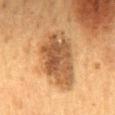workup: no biopsy performed (imaged during a skin exam)
automated metrics: an area of roughly 26 mm², an outline eccentricity of about 0.8 (0 = round, 1 = elongated), and a symmetry-axis asymmetry near 0.2; a within-lesion color-variation index near 5.5/10 and peripheral color asymmetry of about 2; a nevus-likeness score of about 20/100 and a detector confidence of about 100 out of 100 that the crop contains a lesion
lighting: cross-polarized illumination
lesion size: about 7.5 mm
acquisition: ~15 mm crop, total-body skin-cancer survey
subject: male, approximately 60 years of age
site: the mid back